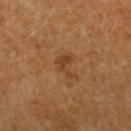Part of a total-body skin-imaging series; this lesion was reviewed on a skin check and was not flagged for biopsy.
A 15 mm crop from a total-body photograph taken for skin-cancer surveillance.
Automated image analysis of the tile measured an area of roughly 4 mm², an outline eccentricity of about 0.8 (0 = round, 1 = elongated), and two-axis asymmetry of about 0.7. The analysis additionally found a detector confidence of about 100 out of 100 that the crop contains a lesion.
On the left forearm.
A female subject, in their mid-50s.
The lesion's longest dimension is about 3 mm.
Captured under cross-polarized illumination.